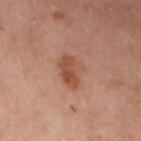This lesion was catalogued during total-body skin photography and was not selected for biopsy. A female subject, in their mid- to late 50s. This is a cross-polarized tile. About 4 mm across. This image is a 15 mm lesion crop taken from a total-body photograph. The lesion is on the left forearm.